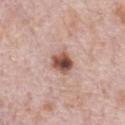Q: Was a biopsy performed?
A: catalogued during a skin exam; not biopsied
Q: What are the patient's age and sex?
A: male, in their 70s
Q: What lighting was used for the tile?
A: white-light
Q: What kind of image is this?
A: ~15 mm tile from a whole-body skin photo
Q: Lesion location?
A: the chest
Q: Lesion size?
A: ~3 mm (longest diameter)